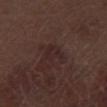follow-up: total-body-photography surveillance lesion; no biopsy | location: the left thigh | subject: male, approximately 70 years of age | image: ~15 mm tile from a whole-body skin photo.